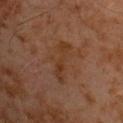notes = total-body-photography surveillance lesion; no biopsy
subject = male, about 60 years old
image source = ~15 mm crop, total-body skin-cancer survey
lighting = cross-polarized illumination
lesion size = ~5 mm (longest diameter)
site = the chest